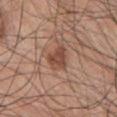biopsy status: imaged on a skin check; not biopsied
subject: male, in their 70s
location: the chest
image source: ~15 mm tile from a whole-body skin photo
tile lighting: white-light illumination